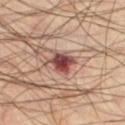Notes:
– workup · total-body-photography surveillance lesion; no biopsy
– acquisition · ~15 mm crop, total-body skin-cancer survey
– location · the left thigh
– patient · male, aged 43–47